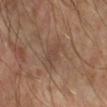automated metrics — a footprint of about 4.5 mm², an eccentricity of roughly 0.75, and two-axis asymmetry of about 0.3; a lesion color around L≈45 a*≈17 b*≈26 in CIELAB, a lesion–skin lightness drop of about 6, and a lesion-to-skin contrast of about 4.5 (normalized; higher = more distinct); a border-irregularity rating of about 3/10, a color-variation rating of about 2/10, and a peripheral color-asymmetry measure near 0.5 | lesion size — about 3 mm | body site — the right forearm | acquisition — ~15 mm tile from a whole-body skin photo.